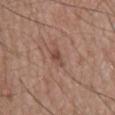The lesion was tiled from a total-body skin photograph and was not biopsied. This is a white-light tile. From the mid back. Approximately 3 mm at its widest. A male subject aged 58 to 62. Automated tile analysis of the lesion measured an area of roughly 3 mm², a shape eccentricity near 0.9, and a symmetry-axis asymmetry near 0.35. It also reported a lesion color around L≈47 a*≈21 b*≈27 in CIELAB and roughly 9 lightness units darker than nearby skin. And it measured border irregularity of about 3.5 on a 0–10 scale and peripheral color asymmetry of about 0.5. This image is a 15 mm lesion crop taken from a total-body photograph.